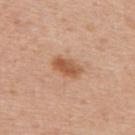Q: Was this lesion biopsied?
A: no biopsy performed (imaged during a skin exam)
Q: Where on the body is the lesion?
A: the upper back
Q: Patient demographics?
A: female, aged 38 to 42
Q: What is the imaging modality?
A: 15 mm crop, total-body photography